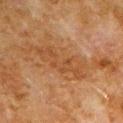Notes:
- follow-up: no biopsy performed (imaged during a skin exam)
- tile lighting: cross-polarized illumination
- anatomic site: the left upper arm
- acquisition: ~15 mm tile from a whole-body skin photo
- automated metrics: a lesion color around L≈38 a*≈19 b*≈32 in CIELAB, roughly 5 lightness units darker than nearby skin, and a normalized border contrast of about 5.5; a border-irregularity rating of about 6.5/10 and peripheral color asymmetry of about 1
- subject: male, aged approximately 80